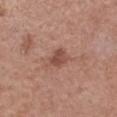notes: total-body-photography surveillance lesion; no biopsy
location: the left forearm
subject: female, roughly 40 years of age
acquisition: 15 mm crop, total-body photography
lesion size: about 3 mm
tile lighting: white-light